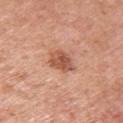| field | value |
|---|---|
| follow-up | no biopsy performed (imaged during a skin exam) |